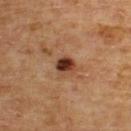  biopsy_status: not biopsied; imaged during a skin examination
  image:
    source: total-body photography crop
    field_of_view_mm: 15
  lesion_size:
    long_diameter_mm_approx: 2.5
  lighting: cross-polarized
  site: upper back
  patient:
    sex: male
    age_approx: 65